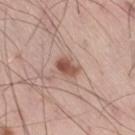Notes:
– follow-up — catalogued during a skin exam; not biopsied
– imaging modality — total-body-photography crop, ~15 mm field of view
– lesion diameter — about 3 mm
– illumination — white-light
– subject — male, about 55 years old
– body site — the right thigh